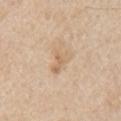Impression:
No biopsy was performed on this lesion — it was imaged during a full skin examination and was not determined to be concerning.
Background:
Located on the right upper arm. A 15 mm crop from a total-body photograph taken for skin-cancer surveillance. The patient is a male roughly 60 years of age. The tile uses white-light illumination.